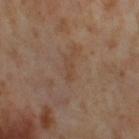– workup · catalogued during a skin exam; not biopsied
– lesion size · about 3 mm
– patient · female, aged 53 to 57
– image source · 15 mm crop, total-body photography
– body site · the leg
– tile lighting · cross-polarized